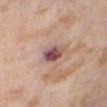Located on the right lower leg.
A female subject in their mid-50s.
Captured under cross-polarized illumination.
The recorded lesion diameter is about 3.5 mm.
A 15 mm crop from a total-body photograph taken for skin-cancer surveillance.